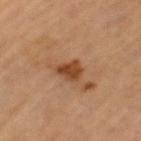biopsy status: imaged on a skin check; not biopsied | illumination: cross-polarized | lesion diameter: ~2.5 mm (longest diameter) | subject: female, aged 68–72 | body site: the left thigh | image: 15 mm crop, total-body photography | image-analysis metrics: a border-irregularity index near 3/10, a color-variation rating of about 3/10, and peripheral color asymmetry of about 1; a classifier nevus-likeness of about 80/100.